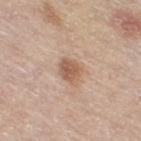Q: Was this lesion biopsied?
A: imaged on a skin check; not biopsied
Q: How was the tile lit?
A: white-light illumination
Q: Who is the patient?
A: female, roughly 65 years of age
Q: Lesion location?
A: the right thigh
Q: What kind of image is this?
A: ~15 mm tile from a whole-body skin photo
Q: Lesion size?
A: ~3 mm (longest diameter)